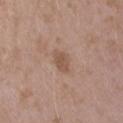* notes — catalogued during a skin exam; not biopsied